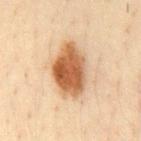Imaged during a routine full-body skin examination; the lesion was not biopsied and no histopathology is available.
The total-body-photography lesion software estimated an eccentricity of roughly 0.8 and two-axis asymmetry of about 0.15. And it measured a lesion color around L≈55 a*≈21 b*≈36 in CIELAB and a lesion-to-skin contrast of about 11 (normalized; higher = more distinct). It also reported a border-irregularity rating of about 1.5/10 and radial color variation of about 1.5. The software also gave a nevus-likeness score of about 100/100 and a detector confidence of about 100 out of 100 that the crop contains a lesion.
A region of skin cropped from a whole-body photographic capture, roughly 15 mm wide.
The patient is a male roughly 35 years of age.
On the chest.